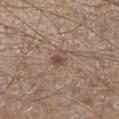biopsy_status: not biopsied; imaged during a skin examination
site: right lower leg
automated_metrics:
  nevus_likeness_0_100: 60
  lesion_detection_confidence_0_100: 100
image:
  source: total-body photography crop
  field_of_view_mm: 15
patient:
  sex: male
  age_approx: 65
lesion_size:
  long_diameter_mm_approx: 2.5